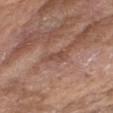Q: Was a biopsy performed?
A: no biopsy performed (imaged during a skin exam)
Q: Automated lesion metrics?
A: a lesion color around L≈47 a*≈21 b*≈26 in CIELAB, about 8 CIELAB-L* units darker than the surrounding skin, and a lesion-to-skin contrast of about 6 (normalized; higher = more distinct); an automated nevus-likeness rating near 0 out of 100 and a detector confidence of about 50 out of 100 that the crop contains a lesion
Q: Patient demographics?
A: female, approximately 75 years of age
Q: Lesion size?
A: ~3 mm (longest diameter)
Q: How was the tile lit?
A: white-light illumination
Q: How was this image acquired?
A: ~15 mm crop, total-body skin-cancer survey
Q: What is the anatomic site?
A: the chest